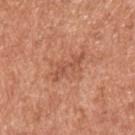Clinical summary: A lesion tile, about 15 mm wide, cut from a 3D total-body photograph. A male patient, aged approximately 55. Located on the back.